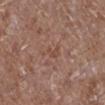Context: About 3 mm across. Cropped from a whole-body photographic skin survey; the tile spans about 15 mm. On the right lower leg. The tile uses white-light illumination. A male patient, aged 43–47.